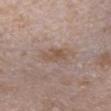{"biopsy_status": "not biopsied; imaged during a skin examination", "image": {"source": "total-body photography crop", "field_of_view_mm": 15}, "lighting": "white-light", "site": "chest", "lesion_size": {"long_diameter_mm_approx": 3.0}, "patient": {"sex": "female", "age_approx": 45}}